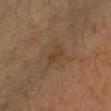This lesion was catalogued during total-body skin photography and was not selected for biopsy.